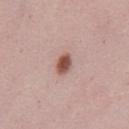The lesion was photographed on a routine skin check and not biopsied; there is no pathology result.
A male subject, aged 48–52.
A close-up tile cropped from a whole-body skin photograph, about 15 mm across.
On the chest.
Measured at roughly 2.5 mm in maximum diameter.